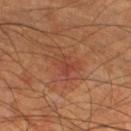Assessment:
Recorded during total-body skin imaging; not selected for excision or biopsy.
Image and clinical context:
This is a cross-polarized tile. The lesion is located on the right lower leg. A 15 mm close-up tile from a total-body photography series done for melanoma screening. A male subject aged approximately 65. Approximately 3.5 mm at its widest.